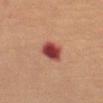Clinical impression:
No biopsy was performed on this lesion — it was imaged during a full skin examination and was not determined to be concerning.
Image and clinical context:
The lesion is located on the leg. Captured under cross-polarized illumination. Longest diameter approximately 3 mm. A female patient aged around 70. A close-up tile cropped from a whole-body skin photograph, about 15 mm across.